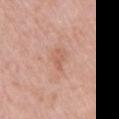biopsy status = imaged on a skin check; not biopsied | lesion diameter = about 2.5 mm | imaging modality = 15 mm crop, total-body photography | lighting = white-light | patient = female, aged approximately 65 | body site = the chest.